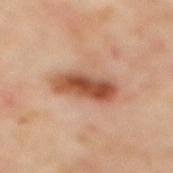A female patient, aged around 60. Cropped from a whole-body photographic skin survey; the tile spans about 15 mm. The lesion is on the back.